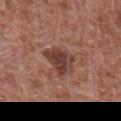biopsy status = catalogued during a skin exam; not biopsied | image = ~15 mm crop, total-body skin-cancer survey | patient = male, about 65 years old | anatomic site = the abdomen.